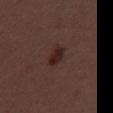Imaged during a routine full-body skin examination; the lesion was not biopsied and no histopathology is available. An algorithmic analysis of the crop reported a footprint of about 3.5 mm², an eccentricity of roughly 0.85, and a symmetry-axis asymmetry near 0.25. And it measured a border-irregularity rating of about 2.5/10, a within-lesion color-variation index near 2.5/10, and a peripheral color-asymmetry measure near 1. And it measured a lesion-detection confidence of about 100/100. The tile uses white-light illumination. Located on the back. A male patient, roughly 30 years of age. A roughly 15 mm field-of-view crop from a total-body skin photograph. Measured at roughly 3 mm in maximum diameter.